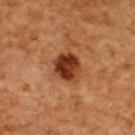notes = imaged on a skin check; not biopsied | automated metrics = a classifier nevus-likeness of about 70/100 and a detector confidence of about 100 out of 100 that the crop contains a lesion | lesion size = ~3.5 mm (longest diameter) | imaging modality = 15 mm crop, total-body photography | site = the upper back | patient = male, about 60 years old | illumination = cross-polarized.